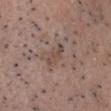  image:
    source: total-body photography crop
    field_of_view_mm: 15
  automated_metrics:
    area_mm2_approx: 3.0
    eccentricity: 0.9
    shape_asymmetry: 0.25
    cielab_L: 47
    cielab_a: 16
    cielab_b: 23
    vs_skin_darker_L: 7.0
    vs_skin_contrast_norm: 6.0
    border_irregularity_0_10: 3.0
    peripheral_color_asymmetry: 0.0
  patient:
    sex: male
    age_approx: 50
  site: head or neck
  lighting: white-light